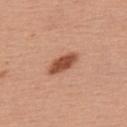TBP lesion metrics = a symmetry-axis asymmetry near 0.25 | image = 15 mm crop, total-body photography | patient = male, aged around 60 | site = the upper back.